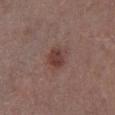This lesion was catalogued during total-body skin photography and was not selected for biopsy. The tile uses white-light illumination. A 15 mm close-up extracted from a 3D total-body photography capture. The lesion is located on the left lower leg. A male subject aged 68–72. The lesion's longest dimension is about 3 mm.